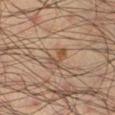Recorded during total-body skin imaging; not selected for excision or biopsy. Measured at roughly 3 mm in maximum diameter. A male subject, aged around 35. From the right lower leg. A 15 mm close-up tile from a total-body photography series done for melanoma screening.